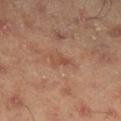<tbp_lesion>
  <biopsy_status>not biopsied; imaged during a skin examination</biopsy_status>
  <patient>
    <sex>male</sex>
    <age_approx>45</age_approx>
  </patient>
  <lesion_size>
    <long_diameter_mm_approx>2.5</long_diameter_mm_approx>
  </lesion_size>
  <automated_metrics>
    <cielab_L>48</cielab_L>
    <cielab_a>22</cielab_a>
    <cielab_b>30</cielab_b>
    <vs_skin_darker_L>7.0</vs_skin_darker_L>
    <vs_skin_contrast_norm>5.5</vs_skin_contrast_norm>
    <peripheral_color_asymmetry>0.5</peripheral_color_asymmetry>
    <nevus_likeness_0_100>0</nevus_likeness_0_100>
    <lesion_detection_confidence_0_100>100</lesion_detection_confidence_0_100>
  </automated_metrics>
  <image>
    <source>total-body photography crop</source>
    <field_of_view_mm>15</field_of_view_mm>
  </image>
  <site>right lower leg</site>
</tbp_lesion>